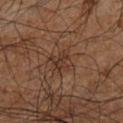workup = imaged on a skin check; not biopsied | tile lighting = cross-polarized | subject = male, in their 60s | lesion diameter = ~3 mm (longest diameter) | acquisition = ~15 mm crop, total-body skin-cancer survey | anatomic site = the left lower leg.